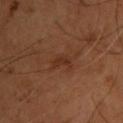{"biopsy_status": "not biopsied; imaged during a skin examination", "patient": {"sex": "male", "age_approx": 55}, "automated_metrics": {"area_mm2_approx": 3.0, "eccentricity": 0.85, "vs_skin_contrast_norm": 6.0, "nevus_likeness_0_100": 0, "lesion_detection_confidence_0_100": 100}, "site": "upper back", "image": {"source": "total-body photography crop", "field_of_view_mm": 15}, "lighting": "cross-polarized", "lesion_size": {"long_diameter_mm_approx": 2.5}}